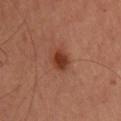Assessment:
No biopsy was performed on this lesion — it was imaged during a full skin examination and was not determined to be concerning.